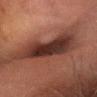<tbp_lesion>
  <biopsy_status>not biopsied; imaged during a skin examination</biopsy_status>
  <patient>
    <sex>male</sex>
    <age_approx>65</age_approx>
  </patient>
  <site>head or neck</site>
  <image>
    <source>total-body photography crop</source>
    <field_of_view_mm>15</field_of_view_mm>
  </image>
  <automated_metrics>
    <cielab_L>26</cielab_L>
    <cielab_a>18</cielab_a>
    <cielab_b>20</cielab_b>
    <vs_skin_darker_L>12.0</vs_skin_darker_L>
    <vs_skin_contrast_norm>12.5</vs_skin_contrast_norm>
    <border_irregularity_0_10>4.5</border_irregularity_0_10>
    <color_variation_0_10>5.0</color_variation_0_10>
  </automated_metrics>
  <lesion_size>
    <long_diameter_mm_approx>9.5</long_diameter_mm_approx>
  </lesion_size>
</tbp_lesion>